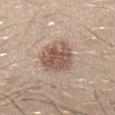Q: Was a biopsy performed?
A: total-body-photography surveillance lesion; no biopsy
Q: What is the lesion's diameter?
A: ≈5 mm
Q: What lighting was used for the tile?
A: white-light
Q: What is the anatomic site?
A: the right upper arm
Q: Patient demographics?
A: male, aged 28–32
Q: How was this image acquired?
A: 15 mm crop, total-body photography
Q: What did automated image analysis measure?
A: a lesion area of about 15 mm², a shape eccentricity near 0.6, and a symmetry-axis asymmetry near 0.2